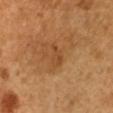Impression: This lesion was catalogued during total-body skin photography and was not selected for biopsy. Image and clinical context: A male patient aged 48–52. Measured at roughly 3 mm in maximum diameter. A 15 mm close-up tile from a total-body photography series done for melanoma screening. From the arm. This is a cross-polarized tile.